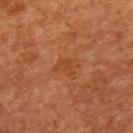{
  "biopsy_status": "not biopsied; imaged during a skin examination",
  "patient": {
    "sex": "female",
    "age_approx": 65
  },
  "lesion_size": {
    "long_diameter_mm_approx": 2.5
  },
  "image": {
    "source": "total-body photography crop",
    "field_of_view_mm": 15
  },
  "site": "left forearm",
  "lighting": "cross-polarized"
}